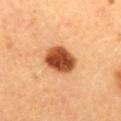Impression: No biopsy was performed on this lesion — it was imaged during a full skin examination and was not determined to be concerning. Acquisition and patient details: On the mid back. A female patient approximately 35 years of age. Longest diameter approximately 4.5 mm. The tile uses cross-polarized illumination. A lesion tile, about 15 mm wide, cut from a 3D total-body photograph.